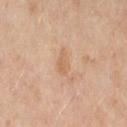Clinical impression: The lesion was tiled from a total-body skin photograph and was not biopsied. Background: The subject is a female in their 50s. The tile uses cross-polarized illumination. A roughly 15 mm field-of-view crop from a total-body skin photograph. On the right thigh. Measured at roughly 2.5 mm in maximum diameter.